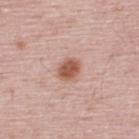The lesion was photographed on a routine skin check and not biopsied; there is no pathology result.
The lesion is on the upper back.
The recorded lesion diameter is about 3 mm.
A 15 mm close-up tile from a total-body photography series done for melanoma screening.
The subject is a male aged around 50.
Automated tile analysis of the lesion measured a footprint of about 5.5 mm² and an outline eccentricity of about 0.6 (0 = round, 1 = elongated). And it measured an automated nevus-likeness rating near 100 out of 100.